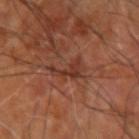An algorithmic analysis of the crop reported a footprint of about 6.5 mm², a shape eccentricity near 0.9, and a symmetry-axis asymmetry near 0.5. The analysis additionally found a color-variation rating of about 2.5/10. The software also gave a nevus-likeness score of about 0/100 and lesion-presence confidence of about 70/100. A subject in their mid-60s. The lesion is located on the right upper arm. Cropped from a whole-body photographic skin survey; the tile spans about 15 mm.